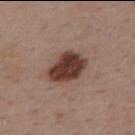Clinical impression:
Captured during whole-body skin photography for melanoma surveillance; the lesion was not biopsied.
Acquisition and patient details:
A female subject aged approximately 45. The lesion is on the mid back. A close-up tile cropped from a whole-body skin photograph, about 15 mm across.